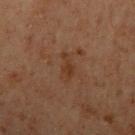Assessment: No biopsy was performed on this lesion — it was imaged during a full skin examination and was not determined to be concerning. Context: From the left upper arm. The patient is a male aged 58 to 62. A roughly 15 mm field-of-view crop from a total-body skin photograph.